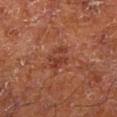<lesion>
  <biopsy_status>not biopsied; imaged during a skin examination</biopsy_status>
  <site>left lower leg</site>
  <lesion_size>
    <long_diameter_mm_approx>3.0</long_diameter_mm_approx>
  </lesion_size>
  <image>
    <source>total-body photography crop</source>
    <field_of_view_mm>15</field_of_view_mm>
  </image>
  <patient>
    <sex>male</sex>
    <age_approx>65</age_approx>
  </patient>
  <lighting>cross-polarized</lighting>
  <automated_metrics>
    <area_mm2_approx>6.0</area_mm2_approx>
    <eccentricity>0.55</eccentricity>
    <shape_asymmetry>0.3</shape_asymmetry>
  </automated_metrics>
</lesion>